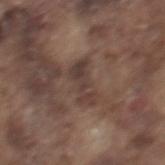follow-up = catalogued during a skin exam; not biopsied | subject = male, approximately 75 years of age | site = the mid back | image source = ~15 mm tile from a whole-body skin photo.